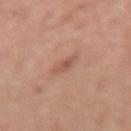• follow-up · total-body-photography surveillance lesion; no biopsy
• size · about 2.5 mm
• site · the right forearm
• image source · ~15 mm crop, total-body skin-cancer survey
• TBP lesion metrics · an average lesion color of about L≈55 a*≈23 b*≈29 (CIELAB), roughly 8 lightness units darker than nearby skin, and a normalized lesion–skin contrast near 5.5
• subject · female, approximately 40 years of age